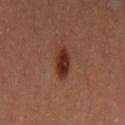Clinical impression: This lesion was catalogued during total-body skin photography and was not selected for biopsy. Clinical summary: The lesion is located on the left upper arm. An algorithmic analysis of the crop reported an area of roughly 6 mm². And it measured an average lesion color of about L≈32 a*≈23 b*≈29 (CIELAB), roughly 12 lightness units darker than nearby skin, and a normalized lesion–skin contrast near 11. It also reported a border-irregularity index near 2.5/10 and a peripheral color-asymmetry measure near 1. It also reported a lesion-detection confidence of about 100/100. The tile uses cross-polarized illumination. The recorded lesion diameter is about 3.5 mm. A female subject, roughly 35 years of age. A close-up tile cropped from a whole-body skin photograph, about 15 mm across.